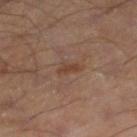Imaged during a routine full-body skin examination; the lesion was not biopsied and no histopathology is available.
A 15 mm close-up tile from a total-body photography series done for melanoma screening.
The lesion's longest dimension is about 2.5 mm.
An algorithmic analysis of the crop reported border irregularity of about 2.5 on a 0–10 scale, a within-lesion color-variation index near 1/10, and radial color variation of about 0.5.
The subject is a male aged around 55.
This is a cross-polarized tile.
The lesion is on the right lower leg.